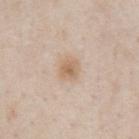Findings:
- workup · imaged on a skin check; not biopsied
- anatomic site · the chest
- lesion diameter · about 2.5 mm
- imaging modality · ~15 mm tile from a whole-body skin photo
- lighting · white-light illumination
- automated metrics · a lesion color around L≈64 a*≈16 b*≈31 in CIELAB and a lesion-to-skin contrast of about 6.5 (normalized; higher = more distinct); an automated nevus-likeness rating near 65 out of 100 and a detector confidence of about 100 out of 100 that the crop contains a lesion
- patient · male, aged approximately 60